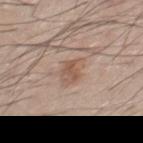| key | value |
|---|---|
| follow-up | imaged on a skin check; not biopsied |
| image | ~15 mm tile from a whole-body skin photo |
| TBP lesion metrics | a within-lesion color-variation index near 4/10 and radial color variation of about 1.5 |
| lesion size | ~3.5 mm (longest diameter) |
| tile lighting | white-light |
| patient | male, approximately 45 years of age |
| site | the chest |